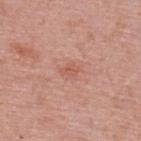{"biopsy_status": "not biopsied; imaged during a skin examination"}